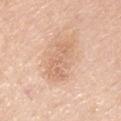Assessment:
Recorded during total-body skin imaging; not selected for excision or biopsy.
Context:
Cropped from a total-body skin-imaging series; the visible field is about 15 mm. The total-body-photography lesion software estimated internal color variation of about 2.5 on a 0–10 scale and a peripheral color-asymmetry measure near 1. From the chest. This is a white-light tile. The subject is a male aged 43 to 47.